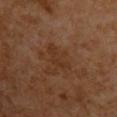Q: Is there a histopathology result?
A: total-body-photography surveillance lesion; no biopsy
Q: What kind of image is this?
A: 15 mm crop, total-body photography
Q: Lesion location?
A: the back
Q: How was the tile lit?
A: cross-polarized illumination
Q: Who is the patient?
A: male, aged 58–62
Q: How large is the lesion?
A: about 3.5 mm